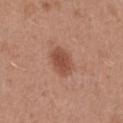Q: Was this lesion biopsied?
A: total-body-photography surveillance lesion; no biopsy
Q: How large is the lesion?
A: about 3.5 mm
Q: How was this image acquired?
A: ~15 mm crop, total-body skin-cancer survey
Q: What is the anatomic site?
A: the left thigh
Q: Patient demographics?
A: female, approximately 40 years of age